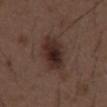No biopsy was performed on this lesion — it was imaged during a full skin examination and was not determined to be concerning. From the abdomen. Cropped from a whole-body photographic skin survey; the tile spans about 15 mm. The total-body-photography lesion software estimated an area of roughly 12 mm², an outline eccentricity of about 0.85 (0 = round, 1 = elongated), and two-axis asymmetry of about 0.2. The analysis additionally found a lesion color around L≈27 a*≈16 b*≈20 in CIELAB and roughly 10 lightness units darker than nearby skin. It also reported an automated nevus-likeness rating near 90 out of 100 and a detector confidence of about 100 out of 100 that the crop contains a lesion. A male subject, aged approximately 50.